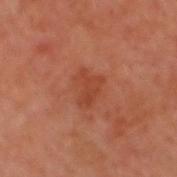biopsy_status: not biopsied; imaged during a skin examination
patient:
  sex: male
  age_approx: 50
lighting: cross-polarized
automated_metrics:
  cielab_L: 33
  cielab_a: 23
  cielab_b: 27
  vs_skin_darker_L: 5.0
  vs_skin_contrast_norm: 5.5
  color_variation_0_10: 1.5
  peripheral_color_asymmetry: 0.5
lesion_size:
  long_diameter_mm_approx: 3.5
image:
  source: total-body photography crop
  field_of_view_mm: 15
site: head or neck